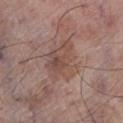Q: Was a biopsy performed?
A: catalogued during a skin exam; not biopsied
Q: What kind of image is this?
A: ~15 mm crop, total-body skin-cancer survey
Q: What is the anatomic site?
A: the left lower leg
Q: What did automated image analysis measure?
A: a footprint of about 15 mm², an outline eccentricity of about 0.6 (0 = round, 1 = elongated), and two-axis asymmetry of about 0.3; an automated nevus-likeness rating near 0 out of 100 and lesion-presence confidence of about 100/100
Q: How large is the lesion?
A: about 4.5 mm
Q: Illumination type?
A: white-light
Q: Patient demographics?
A: male, aged 68–72